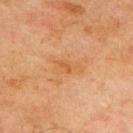Cropped from a whole-body photographic skin survey; the tile spans about 15 mm. The lesion is on the upper back. About 4 mm across. A male subject, aged approximately 70. This is a cross-polarized tile.